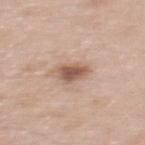{
  "biopsy_status": "not biopsied; imaged during a skin examination",
  "image": {
    "source": "total-body photography crop",
    "field_of_view_mm": 15
  },
  "site": "upper back",
  "patient": {
    "sex": "male",
    "age_approx": 60
  },
  "lighting": "white-light",
  "lesion_size": {
    "long_diameter_mm_approx": 3.0
  }
}